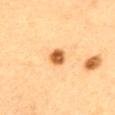Case summary:
* follow-up: imaged on a skin check; not biopsied
* automated metrics: a lesion color around L≈57 a*≈24 b*≈43 in CIELAB, about 17 CIELAB-L* units darker than the surrounding skin, and a normalized lesion–skin contrast near 11
* lesion size: ≈2.5 mm
* subject: female, aged 28 to 32
* lighting: cross-polarized illumination
* acquisition: 15 mm crop, total-body photography
* body site: the upper back